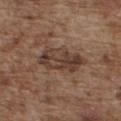{
  "biopsy_status": "not biopsied; imaged during a skin examination",
  "patient": {
    "sex": "male",
    "age_approx": 75
  },
  "image": {
    "source": "total-body photography crop",
    "field_of_view_mm": 15
  },
  "automated_metrics": {
    "cielab_L": 39,
    "cielab_a": 17,
    "cielab_b": 24,
    "vs_skin_darker_L": 10.0,
    "vs_skin_contrast_norm": 8.5
  },
  "site": "chest",
  "lesion_size": {
    "long_diameter_mm_approx": 6.0
  }
}